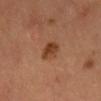The lesion was photographed on a routine skin check and not biopsied; there is no pathology result. A male subject, aged 53 to 57. Cropped from a total-body skin-imaging series; the visible field is about 15 mm. From the mid back. The tile uses cross-polarized illumination. Longest diameter approximately 2.5 mm.